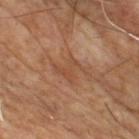| feature | finding |
|---|---|
| illumination | cross-polarized |
| image source | ~15 mm crop, total-body skin-cancer survey |
| image-analysis metrics | a border-irregularity index near 7.5/10 and radial color variation of about 1; a classifier nevus-likeness of about 0/100 and a lesion-detection confidence of about 90/100 |
| patient | male, aged around 55 |
| site | the chest |
| size | ≈4 mm |